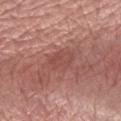Q: Was this lesion biopsied?
A: total-body-photography surveillance lesion; no biopsy
Q: What kind of image is this?
A: total-body-photography crop, ~15 mm field of view
Q: What is the anatomic site?
A: the right forearm
Q: Who is the patient?
A: male, roughly 60 years of age
Q: What lighting was used for the tile?
A: white-light
Q: What did automated image analysis measure?
A: an area of roughly 4.5 mm², an eccentricity of roughly 0.85, and a shape-asymmetry score of about 0.4 (0 = symmetric); an automated nevus-likeness rating near 0 out of 100
Q: What is the lesion's diameter?
A: about 3.5 mm